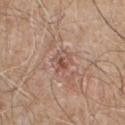Imaged during a routine full-body skin examination; the lesion was not biopsied and no histopathology is available.
Imaged with white-light lighting.
Located on the front of the torso.
A 15 mm close-up extracted from a 3D total-body photography capture.
Longest diameter approximately 2.5 mm.
A male patient, roughly 55 years of age.
An algorithmic analysis of the crop reported a footprint of about 3.5 mm², a shape eccentricity near 0.55, and two-axis asymmetry of about 0.45. The software also gave a border-irregularity rating of about 4.5/10 and peripheral color asymmetry of about 1.